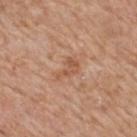notes: no biopsy performed (imaged during a skin exam) | body site: the mid back | lesion diameter: ~3.5 mm (longest diameter) | imaging modality: 15 mm crop, total-body photography | patient: male, aged 58 to 62.